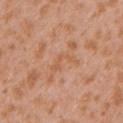biopsy status: catalogued during a skin exam; not biopsied | image-analysis metrics: a lesion color around L≈58 a*≈23 b*≈35 in CIELAB, roughly 5 lightness units darker than nearby skin, and a normalized border contrast of about 5 | lighting: white-light | image: 15 mm crop, total-body photography | subject: female, approximately 30 years of age | site: the left upper arm.